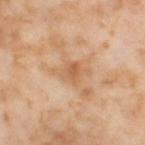No biopsy was performed on this lesion — it was imaged during a full skin examination and was not determined to be concerning.
Imaged with cross-polarized lighting.
Approximately 3.5 mm at its widest.
A female subject aged 53–57.
The lesion is located on the left thigh.
Cropped from a total-body skin-imaging series; the visible field is about 15 mm.